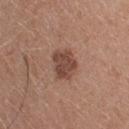biopsy status = total-body-photography surveillance lesion; no biopsy | patient = female, aged 38–42 | image = ~15 mm crop, total-body skin-cancer survey | site = the upper back.